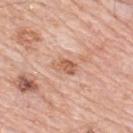Recorded during total-body skin imaging; not selected for excision or biopsy. A male subject aged approximately 80. Cropped from a total-body skin-imaging series; the visible field is about 15 mm. From the upper back. Imaged with white-light lighting. The total-body-photography lesion software estimated an average lesion color of about L≈60 a*≈24 b*≈32 (CIELAB) and a lesion–skin lightness drop of about 10.